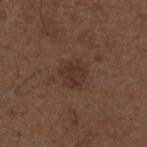biopsy status = catalogued during a skin exam; not biopsied
subject = male, aged 48 to 52
site = the upper back
image source = ~15 mm tile from a whole-body skin photo